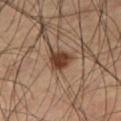No biopsy was performed on this lesion — it was imaged during a full skin examination and was not determined to be concerning. The lesion is located on the right thigh. A male subject aged approximately 60. Measured at roughly 3 mm in maximum diameter. A region of skin cropped from a whole-body photographic capture, roughly 15 mm wide.